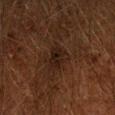The lesion was photographed on a routine skin check and not biopsied; there is no pathology result. Located on the left forearm. A 15 mm close-up extracted from a 3D total-body photography capture. A male subject aged 58–62. Measured at roughly 3 mm in maximum diameter.